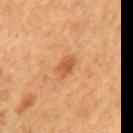Impression: The lesion was tiled from a total-body skin photograph and was not biopsied. Background: The lesion-visualizer software estimated roughly 10 lightness units darker than nearby skin and a normalized border contrast of about 7. Approximately 2.5 mm at its widest. A male subject, aged 63 to 67. A 15 mm close-up tile from a total-body photography series done for melanoma screening. From the mid back.